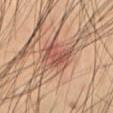notes: total-body-photography surveillance lesion; no biopsy | subject: male, aged around 55 | imaging modality: total-body-photography crop, ~15 mm field of view | anatomic site: the back | tile lighting: cross-polarized | diameter: about 4.5 mm.